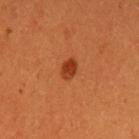Part of a total-body skin-imaging series; this lesion was reviewed on a skin check and was not flagged for biopsy. Automated tile analysis of the lesion measured a footprint of about 4 mm² and a shape-asymmetry score of about 0.2 (0 = symmetric). It also reported an average lesion color of about L≈38 a*≈31 b*≈39 (CIELAB), roughly 10 lightness units darker than nearby skin, and a lesion-to-skin contrast of about 8.5 (normalized; higher = more distinct). And it measured an automated nevus-likeness rating near 100 out of 100 and a detector confidence of about 100 out of 100 that the crop contains a lesion. Measured at roughly 2.5 mm in maximum diameter. A female patient aged around 30. On the left upper arm. Cropped from a whole-body photographic skin survey; the tile spans about 15 mm. Captured under cross-polarized illumination.